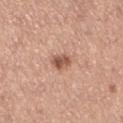Captured during whole-body skin photography for melanoma surveillance; the lesion was not biopsied. Located on the leg. The total-body-photography lesion software estimated a mean CIELAB color near L≈55 a*≈22 b*≈29 and about 13 CIELAB-L* units darker than the surrounding skin. The analysis additionally found border irregularity of about 2.5 on a 0–10 scale, internal color variation of about 4 on a 0–10 scale, and peripheral color asymmetry of about 1.5. This image is a 15 mm lesion crop taken from a total-body photograph. This is a white-light tile. Measured at roughly 2.5 mm in maximum diameter. A female patient, in their mid-60s.